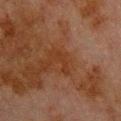<case>
  <biopsy_status>not biopsied; imaged during a skin examination</biopsy_status>
  <site>back</site>
  <automated_metrics>
    <cielab_L>28</cielab_L>
    <cielab_a>19</cielab_a>
    <cielab_b>28</cielab_b>
    <vs_skin_darker_L>4.0</vs_skin_darker_L>
  </automated_metrics>
  <lesion_size>
    <long_diameter_mm_approx>3.0</long_diameter_mm_approx>
  </lesion_size>
  <patient>
    <sex>male</sex>
    <age_approx>80</age_approx>
  </patient>
  <image>
    <source>total-body photography crop</source>
    <field_of_view_mm>15</field_of_view_mm>
  </image>
  <lighting>cross-polarized</lighting>
</case>